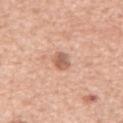Imaged during a routine full-body skin examination; the lesion was not biopsied and no histopathology is available. The tile uses white-light illumination. Approximately 2.5 mm at its widest. A region of skin cropped from a whole-body photographic capture, roughly 15 mm wide. The lesion is on the abdomen. A male subject, in their mid-60s.